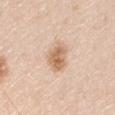{
  "biopsy_status": "not biopsied; imaged during a skin examination",
  "image": {
    "source": "total-body photography crop",
    "field_of_view_mm": 15
  },
  "lesion_size": {
    "long_diameter_mm_approx": 3.0
  },
  "patient": {
    "sex": "male",
    "age_approx": 45
  },
  "site": "right upper arm"
}